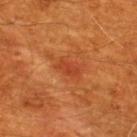Clinical impression:
Part of a total-body skin-imaging series; this lesion was reviewed on a skin check and was not flagged for biopsy.